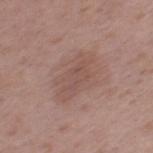This lesion was catalogued during total-body skin photography and was not selected for biopsy.
A 15 mm close-up extracted from a 3D total-body photography capture.
Captured under white-light illumination.
Automated image analysis of the tile measured about 6 CIELAB-L* units darker than the surrounding skin and a normalized lesion–skin contrast near 4.5. It also reported a classifier nevus-likeness of about 0/100 and lesion-presence confidence of about 100/100.
From the left thigh.
A female patient, aged 53–57.
The lesion's longest dimension is about 5.5 mm.